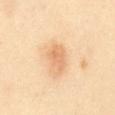Impression: The lesion was photographed on a routine skin check and not biopsied; there is no pathology result. Clinical summary: A region of skin cropped from a whole-body photographic capture, roughly 15 mm wide. Automated tile analysis of the lesion measured an area of roughly 6 mm², an eccentricity of roughly 0.75, and a symmetry-axis asymmetry near 0.25. And it measured an average lesion color of about L≈73 a*≈22 b*≈41 (CIELAB), roughly 9 lightness units darker than nearby skin, and a lesion-to-skin contrast of about 5.5 (normalized; higher = more distinct). And it measured a border-irregularity rating of about 2.5/10, a within-lesion color-variation index near 3/10, and peripheral color asymmetry of about 1. From the abdomen. The patient is a female aged 58–62. The tile uses cross-polarized illumination. Longest diameter approximately 3.5 mm.